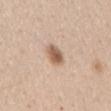| feature | finding |
|---|---|
| imaging modality | 15 mm crop, total-body photography |
| illumination | white-light |
| body site | the right upper arm |
| lesion size | about 2.5 mm |
| patient | female, aged 38 to 42 |